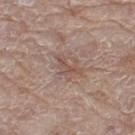Q: Was a biopsy performed?
A: catalogued during a skin exam; not biopsied
Q: Lesion location?
A: the leg
Q: What are the patient's age and sex?
A: male, roughly 80 years of age
Q: What is the imaging modality?
A: 15 mm crop, total-body photography
Q: Illumination type?
A: white-light illumination
Q: Automated lesion metrics?
A: an area of roughly 3.5 mm² and an outline eccentricity of about 0.9 (0 = round, 1 = elongated); an average lesion color of about L≈51 a*≈18 b*≈24 (CIELAB) and roughly 7 lightness units darker than nearby skin; internal color variation of about 0 on a 0–10 scale and peripheral color asymmetry of about 0